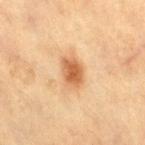{
  "biopsy_status": "not biopsied; imaged during a skin examination",
  "lesion_size": {
    "long_diameter_mm_approx": 3.5
  },
  "image": {
    "source": "total-body photography crop",
    "field_of_view_mm": 15
  },
  "automated_metrics": {
    "vs_skin_darker_L": 12.0,
    "nevus_likeness_0_100": 100,
    "lesion_detection_confidence_0_100": 100
  },
  "site": "right thigh",
  "patient": {
    "sex": "female",
    "age_approx": 40
  },
  "lighting": "cross-polarized"
}